The lesion is located on the left forearm. Approximately 1 mm at its widest. A lesion tile, about 15 mm wide, cut from a 3D total-body photograph. Captured under cross-polarized illumination. The lesion-visualizer software estimated an outline eccentricity of about 0.75 (0 = round, 1 = elongated) and a shape-asymmetry score of about 0.35 (0 = symmetric). It also reported a mean CIELAB color near L≈38 a*≈23 b*≈23 and roughly 4 lightness units darker than nearby skin — 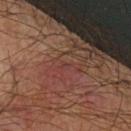• histopathologic diagnosis: a basal cell carcinoma (malignant)